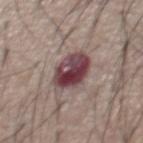This lesion was catalogued during total-body skin photography and was not selected for biopsy. The subject is a male approximately 55 years of age. A 15 mm crop from a total-body photograph taken for skin-cancer surveillance. From the front of the torso.